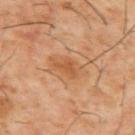workup: total-body-photography surveillance lesion; no biopsy | size: ≈3.5 mm | acquisition: 15 mm crop, total-body photography | anatomic site: the upper back | automated metrics: a lesion-to-skin contrast of about 6.5 (normalized; higher = more distinct); a color-variation rating of about 1/10 and peripheral color asymmetry of about 0.5; a classifier nevus-likeness of about 0/100 and a detector confidence of about 100 out of 100 that the crop contains a lesion | tile lighting: cross-polarized | patient: male, in their 60s.